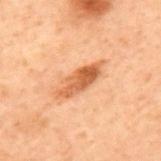Findings:
* workup · imaged on a skin check; not biopsied
* site · the mid back
* diameter · about 5.5 mm
* subject · male, aged 68 to 72
* tile lighting · cross-polarized illumination
* image · 15 mm crop, total-body photography